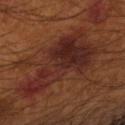The lesion is located on the right forearm.
This is a cross-polarized tile.
A male patient, roughly 55 years of age.
An algorithmic analysis of the crop reported a lesion area of about 32 mm², an outline eccentricity of about 0.85 (0 = round, 1 = elongated), and a symmetry-axis asymmetry near 0.6. The software also gave a nevus-likeness score of about 0/100 and lesion-presence confidence of about 100/100.
A lesion tile, about 15 mm wide, cut from a 3D total-body photograph.